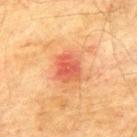Impression: No biopsy was performed on this lesion — it was imaged during a full skin examination and was not determined to be concerning. Clinical summary: The patient is a male roughly 75 years of age. From the mid back. A lesion tile, about 15 mm wide, cut from a 3D total-body photograph. The tile uses cross-polarized illumination.